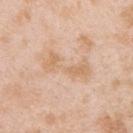<tbp_lesion>
  <biopsy_status>not biopsied; imaged during a skin examination</biopsy_status>
  <lighting>white-light</lighting>
  <patient>
    <sex>male</sex>
    <age_approx>25</age_approx>
  </patient>
  <site>upper back</site>
  <lesion_size>
    <long_diameter_mm_approx>6.0</long_diameter_mm_approx>
  </lesion_size>
  <image>
    <source>total-body photography crop</source>
    <field_of_view_mm>15</field_of_view_mm>
  </image>
</tbp_lesion>